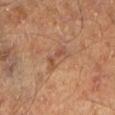Captured during whole-body skin photography for melanoma surveillance; the lesion was not biopsied. Measured at roughly 3.5 mm in maximum diameter. Cropped from a total-body skin-imaging series; the visible field is about 15 mm. A male subject, aged approximately 60. Located on the left lower leg. This is a cross-polarized tile.